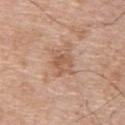The lesion was tiled from a total-body skin photograph and was not biopsied.
The lesion is located on the upper back.
Cropped from a total-body skin-imaging series; the visible field is about 15 mm.
Automated tile analysis of the lesion measured an average lesion color of about L≈57 a*≈20 b*≈31 (CIELAB) and about 9 CIELAB-L* units darker than the surrounding skin. The software also gave an automated nevus-likeness rating near 0 out of 100 and a lesion-detection confidence of about 100/100.
A male subject, in their mid- to late 60s.
Captured under white-light illumination.
Approximately 3.5 mm at its widest.